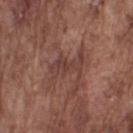Imaged during a routine full-body skin examination; the lesion was not biopsied and no histopathology is available.
The tile uses white-light illumination.
Measured at roughly 5 mm in maximum diameter.
The subject is a male aged approximately 75.
A 15 mm close-up tile from a total-body photography series done for melanoma screening.
On the right upper arm.
Automated tile analysis of the lesion measured an eccentricity of roughly 0.85 and a symmetry-axis asymmetry near 0.45. It also reported an average lesion color of about L≈40 a*≈21 b*≈23 (CIELAB). The analysis additionally found border irregularity of about 7 on a 0–10 scale, internal color variation of about 2.5 on a 0–10 scale, and radial color variation of about 0.5.